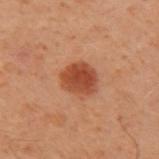No biopsy was performed on this lesion — it was imaged during a full skin examination and was not determined to be concerning.
The patient is a male approximately 50 years of age.
The lesion-visualizer software estimated an area of roughly 9 mm², an outline eccentricity of about 0.5 (0 = round, 1 = elongated), and two-axis asymmetry of about 0.15. The software also gave a border-irregularity index near 1.5/10, internal color variation of about 3 on a 0–10 scale, and peripheral color asymmetry of about 1. And it measured an automated nevus-likeness rating near 100 out of 100 and lesion-presence confidence of about 100/100.
The lesion is located on the left upper arm.
A roughly 15 mm field-of-view crop from a total-body skin photograph.
The recorded lesion diameter is about 3.5 mm.